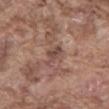Assessment:
This lesion was catalogued during total-body skin photography and was not selected for biopsy.
Image and clinical context:
The total-body-photography lesion software estimated a border-irregularity rating of about 4/10 and internal color variation of about 2.5 on a 0–10 scale. The analysis additionally found an automated nevus-likeness rating near 0 out of 100 and a detector confidence of about 90 out of 100 that the crop contains a lesion. The recorded lesion diameter is about 3 mm. A male subject, in their mid-70s. A 15 mm close-up tile from a total-body photography series done for melanoma screening. Captured under white-light illumination. Located on the abdomen.